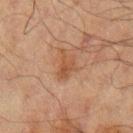Assessment:
The lesion was photographed on a routine skin check and not biopsied; there is no pathology result.
Background:
Longest diameter approximately 3.5 mm. A male patient aged 68–72. Imaged with cross-polarized lighting. Automated image analysis of the tile measured border irregularity of about 4.5 on a 0–10 scale, a color-variation rating of about 2.5/10, and a peripheral color-asymmetry measure near 1. The software also gave a nevus-likeness score of about 10/100 and a detector confidence of about 100 out of 100 that the crop contains a lesion. Located on the left upper arm. A region of skin cropped from a whole-body photographic capture, roughly 15 mm wide.